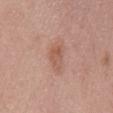| field | value |
|---|---|
| biopsy status | catalogued during a skin exam; not biopsied |
| acquisition | total-body-photography crop, ~15 mm field of view |
| tile lighting | white-light illumination |
| automated metrics | a lesion area of about 4.5 mm², a shape eccentricity near 0.75, and a symmetry-axis asymmetry near 0.35; roughly 8 lightness units darker than nearby skin; a border-irregularity rating of about 3/10 and a color-variation rating of about 3.5/10; a nevus-likeness score of about 30/100 and lesion-presence confidence of about 100/100 |
| patient | male, aged around 70 |
| size | ≈3 mm |
| site | the mid back |